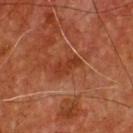Image and clinical context: Measured at roughly 3.5 mm in maximum diameter. The subject is a male aged 53 to 57. From the chest. A 15 mm close-up tile from a total-body photography series done for melanoma screening. Imaged with cross-polarized lighting.